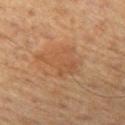A 15 mm close-up tile from a total-body photography series done for melanoma screening.
About 5 mm across.
The patient is a male in their mid- to late 60s.
The total-body-photography lesion software estimated border irregularity of about 6.5 on a 0–10 scale, internal color variation of about 2.5 on a 0–10 scale, and a peripheral color-asymmetry measure near 1. It also reported a lesion-detection confidence of about 100/100.
Imaged with cross-polarized lighting.
On the left thigh.